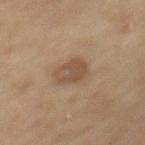A lesion tile, about 15 mm wide, cut from a 3D total-body photograph. Captured under cross-polarized illumination. A female patient approximately 60 years of age. Automated image analysis of the tile measured a lesion-detection confidence of about 100/100. About 3.5 mm across. The lesion is on the mid back.